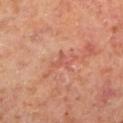Part of a total-body skin-imaging series; this lesion was reviewed on a skin check and was not flagged for biopsy.
Cropped from a total-body skin-imaging series; the visible field is about 15 mm.
Longest diameter approximately 2.5 mm.
A male subject, about 60 years old.
The lesion-visualizer software estimated a lesion color around L≈52 a*≈27 b*≈29 in CIELAB, about 6 CIELAB-L* units darker than the surrounding skin, and a normalized border contrast of about 4.5. And it measured a border-irregularity index near 7/10, internal color variation of about 0 on a 0–10 scale, and a peripheral color-asymmetry measure near 0.
From the left lower leg.